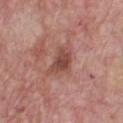• workup — imaged on a skin check; not biopsied
• acquisition — 15 mm crop, total-body photography
• location — the chest
• lesion diameter — about 4 mm
• tile lighting — white-light
• subject — male, in their mid-60s